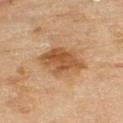patient: female, aged 58 to 62 | lesion size: ~5 mm (longest diameter) | body site: the left thigh | illumination: cross-polarized | image source: total-body-photography crop, ~15 mm field of view | TBP lesion metrics: a footprint of about 16 mm², an eccentricity of roughly 0.6, and two-axis asymmetry of about 0.2; a lesion–skin lightness drop of about 11 and a lesion-to-skin contrast of about 8 (normalized; higher = more distinct); border irregularity of about 2.5 on a 0–10 scale, a color-variation rating of about 4.5/10, and peripheral color asymmetry of about 1.5.